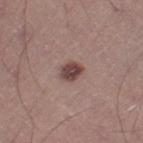Impression:
This lesion was catalogued during total-body skin photography and was not selected for biopsy.
Acquisition and patient details:
A 15 mm crop from a total-body photograph taken for skin-cancer surveillance. A male patient aged 43–47. Longest diameter approximately 2.5 mm. Imaged with white-light lighting. On the left thigh.